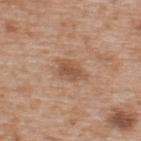Q: What are the patient's age and sex?
A: male, aged around 65
Q: Lesion size?
A: ≈3.5 mm
Q: Where on the body is the lesion?
A: the upper back
Q: Illumination type?
A: white-light illumination
Q: Automated lesion metrics?
A: a lesion area of about 6 mm²; a mean CIELAB color near L≈53 a*≈20 b*≈31, roughly 9 lightness units darker than nearby skin, and a lesion-to-skin contrast of about 6.5 (normalized; higher = more distinct); a border-irregularity rating of about 3/10 and a within-lesion color-variation index near 2/10; a classifier nevus-likeness of about 5/100
Q: What kind of image is this?
A: 15 mm crop, total-body photography